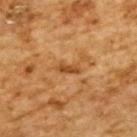This lesion was catalogued during total-body skin photography and was not selected for biopsy. The patient is a male aged approximately 85 or older. This is a cross-polarized tile. On the back. Approximately 3 mm at its widest. Cropped from a total-body skin-imaging series; the visible field is about 15 mm.